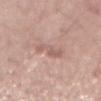No biopsy was performed on this lesion — it was imaged during a full skin examination and was not determined to be concerning. A male patient aged 53 to 57. A close-up tile cropped from a whole-body skin photograph, about 15 mm across. About 3 mm across. Imaged with white-light lighting. The lesion is on the abdomen. The lesion-visualizer software estimated a lesion area of about 5.5 mm², an eccentricity of roughly 0.65, and two-axis asymmetry of about 0.25. And it measured a lesion color around L≈59 a*≈20 b*≈24 in CIELAB, a lesion–skin lightness drop of about 8, and a normalized border contrast of about 5. The analysis additionally found a nevus-likeness score of about 0/100 and a detector confidence of about 100 out of 100 that the crop contains a lesion.